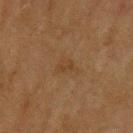Captured during whole-body skin photography for melanoma surveillance; the lesion was not biopsied. A male subject aged 73 to 77. Cropped from a whole-body photographic skin survey; the tile spans about 15 mm. Located on the upper back.